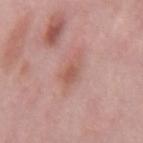notes: imaged on a skin check; not biopsied | automated lesion analysis: an area of roughly 5.5 mm², an outline eccentricity of about 0.8 (0 = round, 1 = elongated), and a shape-asymmetry score of about 0.25 (0 = symmetric); an average lesion color of about L≈57 a*≈23 b*≈27 (CIELAB) and about 8 CIELAB-L* units darker than the surrounding skin; a border-irregularity rating of about 3/10 and a color-variation rating of about 3/10; a classifier nevus-likeness of about 0/100 | image: total-body-photography crop, ~15 mm field of view | lesion size: about 3 mm | subject: male, aged around 55 | anatomic site: the mid back.